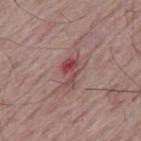Part of a total-body skin-imaging series; this lesion was reviewed on a skin check and was not flagged for biopsy.
Cropped from a whole-body photographic skin survey; the tile spans about 15 mm.
The lesion's longest dimension is about 3.5 mm.
The patient is a male aged approximately 65.
Located on the mid back.
This is a white-light tile.